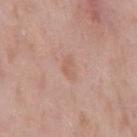Assessment: Imaged during a routine full-body skin examination; the lesion was not biopsied and no histopathology is available. Acquisition and patient details: Captured under white-light illumination. Automated image analysis of the tile measured an eccentricity of roughly 0.85 and a shape-asymmetry score of about 0.4 (0 = symmetric). It also reported a lesion–skin lightness drop of about 6. It also reported an automated nevus-likeness rating near 0 out of 100 and lesion-presence confidence of about 100/100. The patient is a male about 55 years old. A roughly 15 mm field-of-view crop from a total-body skin photograph. The lesion is located on the mid back. The lesion's longest dimension is about 3 mm.